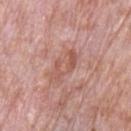{"biopsy_status": "not biopsied; imaged during a skin examination", "patient": {"sex": "male", "age_approx": 70}, "image": {"source": "total-body photography crop", "field_of_view_mm": 15}, "site": "front of the torso", "lesion_size": {"long_diameter_mm_approx": 4.0}, "automated_metrics": {"area_mm2_approx": 6.0, "eccentricity": 0.9, "shape_asymmetry": 0.35, "vs_skin_darker_L": 8.0, "vs_skin_contrast_norm": 6.0, "nevus_likeness_0_100": 0, "lesion_detection_confidence_0_100": 100}, "lighting": "white-light"}